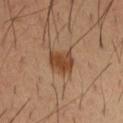biopsy status: total-body-photography surveillance lesion; no biopsy
image: ~15 mm crop, total-body skin-cancer survey
anatomic site: the left forearm
patient: male, aged around 40
lighting: cross-polarized
size: ≈3 mm